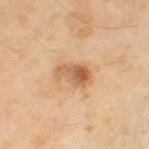Context:
A close-up tile cropped from a whole-body skin photograph, about 15 mm across. The lesion is on the left thigh. Captured under cross-polarized illumination. Measured at roughly 4 mm in maximum diameter. A male patient aged 53–57. The lesion-visualizer software estimated an eccentricity of roughly 0.8 and a shape-asymmetry score of about 0.35 (0 = symmetric). The analysis additionally found a mean CIELAB color near L≈59 a*≈21 b*≈36. The software also gave a lesion-detection confidence of about 100/100.